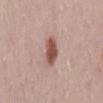No biopsy was performed on this lesion — it was imaged during a full skin examination and was not determined to be concerning.
The lesion is on the mid back.
A 15 mm crop from a total-body photograph taken for skin-cancer surveillance.
Approximately 3.5 mm at its widest.
Captured under white-light illumination.
The patient is a female in their 30s.